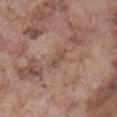{"biopsy_status": "not biopsied; imaged during a skin examination", "lesion_size": {"long_diameter_mm_approx": 2.5}, "image": {"source": "total-body photography crop", "field_of_view_mm": 15}, "patient": {"sex": "male", "age_approx": 75}, "site": "abdomen", "lighting": "white-light", "automated_metrics": {"cielab_L": 49, "cielab_a": 19, "cielab_b": 25, "vs_skin_contrast_norm": 6.0, "border_irregularity_0_10": 3.5, "peripheral_color_asymmetry": 0.0}}